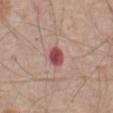notes: no biopsy performed (imaged during a skin exam); body site: the front of the torso; image: ~15 mm crop, total-body skin-cancer survey; patient: male, aged around 80.